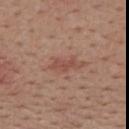The lesion was photographed on a routine skin check and not biopsied; there is no pathology result.
Captured under white-light illumination.
A male subject aged 53–57.
Located on the mid back.
Cropped from a whole-body photographic skin survey; the tile spans about 15 mm.
The lesion's longest dimension is about 3 mm.